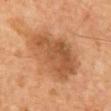Assessment: Captured during whole-body skin photography for melanoma surveillance; the lesion was not biopsied. Background: The patient is a male aged around 60. The lesion is on the chest. The tile uses cross-polarized illumination. A close-up tile cropped from a whole-body skin photograph, about 15 mm across.